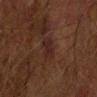| feature | finding |
|---|---|
| biopsy status | no biopsy performed (imaged during a skin exam) |
| lighting | cross-polarized |
| lesion size | about 3 mm |
| subject | male, aged 73 to 77 |
| imaging modality | total-body-photography crop, ~15 mm field of view |
| TBP lesion metrics | about 5 CIELAB-L* units darker than the surrounding skin and a lesion-to-skin contrast of about 7 (normalized; higher = more distinct) |
| anatomic site | the right upper arm |